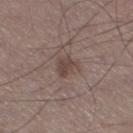– notes · catalogued during a skin exam; not biopsied
– TBP lesion metrics · a lesion area of about 5 mm² and two-axis asymmetry of about 0.35; border irregularity of about 3.5 on a 0–10 scale, internal color variation of about 2 on a 0–10 scale, and radial color variation of about 0.5
– diameter · about 2.5 mm
– imaging modality · 15 mm crop, total-body photography
– subject · male, aged approximately 55
– location · the right thigh